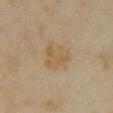workup: catalogued during a skin exam; not biopsied | site: the front of the torso | lesion diameter: ≈4 mm | acquisition: ~15 mm crop, total-body skin-cancer survey | patient: female, aged around 35 | illumination: cross-polarized | automated lesion analysis: a border-irregularity rating of about 3/10, internal color variation of about 3 on a 0–10 scale, and a peripheral color-asymmetry measure near 1.